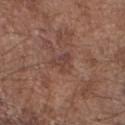notes=imaged on a skin check; not biopsied | automated lesion analysis=peripheral color asymmetry of about 1; a nevus-likeness score of about 0/100 and lesion-presence confidence of about 100/100 | image=~15 mm crop, total-body skin-cancer survey | site=the right forearm | diameter=about 3 mm | illumination=white-light illumination | patient=male, aged around 70.